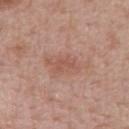Case summary:
* biopsy status — total-body-photography surveillance lesion; no biopsy
* lesion diameter — about 3 mm
* body site — the mid back
* patient — male, aged around 65
* illumination — white-light
* imaging modality — 15 mm crop, total-body photography